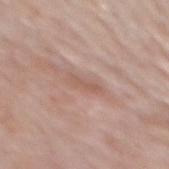Captured during whole-body skin photography for melanoma surveillance; the lesion was not biopsied. This is a white-light tile. The lesion is on the mid back. A male subject aged approximately 55. Automated tile analysis of the lesion measured a lesion area of about 3.5 mm² and an eccentricity of roughly 0.95. And it measured a border-irregularity rating of about 4/10 and a color-variation rating of about 0/10. A close-up tile cropped from a whole-body skin photograph, about 15 mm across.